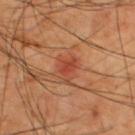<case>
<biopsy_status>not biopsied; imaged during a skin examination</biopsy_status>
<patient>
  <sex>male</sex>
  <age_approx>60</age_approx>
</patient>
<lighting>cross-polarized</lighting>
<image>
  <source>total-body photography crop</source>
  <field_of_view_mm>15</field_of_view_mm>
</image>
<site>upper back</site>
</case>